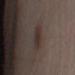Acquisition and patient details: A female patient roughly 20 years of age. This is a white-light tile. Cropped from a whole-body photographic skin survey; the tile spans about 15 mm. The lesion is on the leg. Measured at roughly 3 mm in maximum diameter.